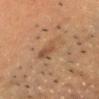Q: Was this lesion biopsied?
A: catalogued during a skin exam; not biopsied
Q: What lighting was used for the tile?
A: cross-polarized
Q: How large is the lesion?
A: ~3.5 mm (longest diameter)
Q: What are the patient's age and sex?
A: male, roughly 60 years of age
Q: What did automated image analysis measure?
A: an area of roughly 7.5 mm², an outline eccentricity of about 0.6 (0 = round, 1 = elongated), and a symmetry-axis asymmetry near 0.2; a lesion color around L≈46 a*≈17 b*≈30 in CIELAB and a normalized border contrast of about 5; border irregularity of about 2.5 on a 0–10 scale, internal color variation of about 4 on a 0–10 scale, and peripheral color asymmetry of about 1.5
Q: Where on the body is the lesion?
A: the head or neck
Q: How was this image acquired?
A: ~15 mm crop, total-body skin-cancer survey